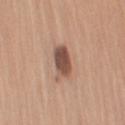No biopsy was performed on this lesion — it was imaged during a full skin examination and was not determined to be concerning.
Approximately 4.5 mm at its widest.
A female subject in their mid- to late 40s.
Captured under white-light illumination.
Located on the left upper arm.
This image is a 15 mm lesion crop taken from a total-body photograph.